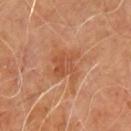Automated tile analysis of the lesion measured an area of roughly 8 mm² and an eccentricity of roughly 0.4. The analysis additionally found a lesion color around L≈49 a*≈25 b*≈35 in CIELAB and a normalized border contrast of about 6. The analysis additionally found a border-irregularity rating of about 4/10, internal color variation of about 3.5 on a 0–10 scale, and a peripheral color-asymmetry measure near 1. It also reported an automated nevus-likeness rating near 15 out of 100 and lesion-presence confidence of about 100/100. Captured under cross-polarized illumination. A male subject roughly 60 years of age. A close-up tile cropped from a whole-body skin photograph, about 15 mm across. The recorded lesion diameter is about 3.5 mm. On the upper back.